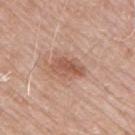Clinical impression: The lesion was photographed on a routine skin check and not biopsied; there is no pathology result. Background: This is a white-light tile. A male patient, aged approximately 75. From the right upper arm. A roughly 15 mm field-of-view crop from a total-body skin photograph. The lesion's longest dimension is about 4 mm. An algorithmic analysis of the crop reported an outline eccentricity of about 0.85 (0 = round, 1 = elongated) and a symmetry-axis asymmetry near 0.2. It also reported an average lesion color of about L≈56 a*≈22 b*≈29 (CIELAB), a lesion–skin lightness drop of about 10, and a normalized border contrast of about 7. The software also gave border irregularity of about 2.5 on a 0–10 scale, a color-variation rating of about 4/10, and peripheral color asymmetry of about 1.5. The analysis additionally found an automated nevus-likeness rating near 20 out of 100 and lesion-presence confidence of about 100/100.